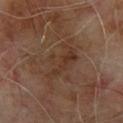Impression: No biopsy was performed on this lesion — it was imaged during a full skin examination and was not determined to be concerning. Background: A male subject, in their mid-60s. The tile uses cross-polarized illumination. A region of skin cropped from a whole-body photographic capture, roughly 15 mm wide. From the back. About 7 mm across.